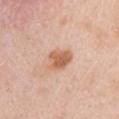Findings:
- biopsy status — catalogued during a skin exam; not biopsied
- tile lighting — white-light illumination
- lesion size — about 3 mm
- imaging modality — 15 mm crop, total-body photography
- patient — female, aged 48–52
- location — the right upper arm